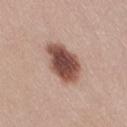| field | value |
|---|---|
| follow-up | no biopsy performed (imaged during a skin exam) |
| anatomic site | the right thigh |
| automated lesion analysis | an average lesion color of about L≈48 a*≈22 b*≈25 (CIELAB) and a normalized lesion–skin contrast near 12.5; a border-irregularity index near 2/10, a within-lesion color-variation index near 4.5/10, and peripheral color asymmetry of about 1 |
| size | ~5 mm (longest diameter) |
| patient | female, in their mid-20s |
| imaging modality | total-body-photography crop, ~15 mm field of view |
| illumination | white-light |